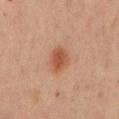{"biopsy_status": "not biopsied; imaged during a skin examination", "patient": {"sex": "male", "age_approx": 70}, "site": "abdomen", "image": {"source": "total-body photography crop", "field_of_view_mm": 15}}